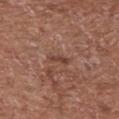biopsy_status: not biopsied; imaged during a skin examination
patient:
  sex: female
  age_approx: 75
lighting: white-light
image:
  source: total-body photography crop
  field_of_view_mm: 15
lesion_size:
  long_diameter_mm_approx: 3.0
site: chest